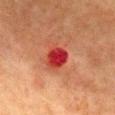Imaged during a routine full-body skin examination; the lesion was not biopsied and no histopathology is available. A female subject aged 48 to 52. Located on the chest. An algorithmic analysis of the crop reported a footprint of about 6 mm² and a symmetry-axis asymmetry near 0.2. The software also gave a border-irregularity index near 1.5/10, internal color variation of about 3.5 on a 0–10 scale, and radial color variation of about 1. Cropped from a total-body skin-imaging series; the visible field is about 15 mm. Approximately 2.5 mm at its widest. The tile uses cross-polarized illumination.